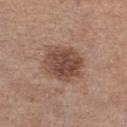| field | value |
|---|---|
| notes | imaged on a skin check; not biopsied |
| diameter | about 5 mm |
| image | total-body-photography crop, ~15 mm field of view |
| subject | female, aged around 45 |
| anatomic site | the front of the torso |
| TBP lesion metrics | an average lesion color of about L≈46 a*≈20 b*≈26 (CIELAB) and a normalized lesion–skin contrast near 9; a nevus-likeness score of about 40/100 and a detector confidence of about 100 out of 100 that the crop contains a lesion |